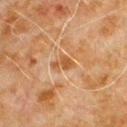Notes:
* workup — total-body-photography surveillance lesion; no biopsy
* diameter — ~2.5 mm (longest diameter)
* site — the chest
* image — ~15 mm tile from a whole-body skin photo
* subject — male, aged 78 to 82
* lighting — cross-polarized illumination
* automated metrics — two-axis asymmetry of about 0.45; a color-variation rating of about 1.5/10 and radial color variation of about 0.5